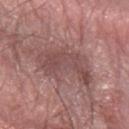* lesion diameter: ≈6.5 mm
* anatomic site: the arm
* tile lighting: white-light
* patient: male, in their 60s
* image: 15 mm crop, total-body photography